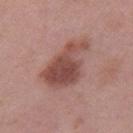This lesion was catalogued during total-body skin photography and was not selected for biopsy. A roughly 15 mm field-of-view crop from a total-body skin photograph. An algorithmic analysis of the crop reported a border-irregularity rating of about 4/10, internal color variation of about 5 on a 0–10 scale, and peripheral color asymmetry of about 1.5. The analysis additionally found an automated nevus-likeness rating near 65 out of 100 and a lesion-detection confidence of about 100/100. Imaged with white-light lighting. Approximately 7 mm at its widest. The lesion is located on the right thigh. The subject is a female roughly 40 years of age.